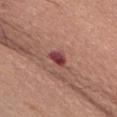Impression: The lesion was photographed on a routine skin check and not biopsied; there is no pathology result. Background: Automated image analysis of the tile measured an average lesion color of about L≈42 a*≈30 b*≈21 (CIELAB), a lesion–skin lightness drop of about 14, and a lesion-to-skin contrast of about 11 (normalized; higher = more distinct). The software also gave border irregularity of about 3 on a 0–10 scale. The analysis additionally found an automated nevus-likeness rating near 0 out of 100 and lesion-presence confidence of about 100/100. A roughly 15 mm field-of-view crop from a total-body skin photograph. On the abdomen. This is a white-light tile. A female patient in their mid- to late 60s.